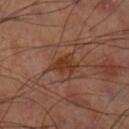Captured during whole-body skin photography for melanoma surveillance; the lesion was not biopsied.
A 15 mm crop from a total-body photograph taken for skin-cancer surveillance.
Located on the right thigh.